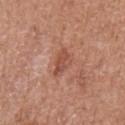Case summary:
– workup: imaged on a skin check; not biopsied
– size: about 3.5 mm
– image source: 15 mm crop, total-body photography
– lighting: white-light
– anatomic site: the left upper arm
– subject: female, aged approximately 55